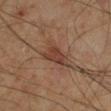A male patient, approximately 70 years of age.
Cropped from a total-body skin-imaging series; the visible field is about 15 mm.
This is a cross-polarized tile.
The lesion is located on the leg.
An algorithmic analysis of the crop reported an outline eccentricity of about 0.9 (0 = round, 1 = elongated). The software also gave border irregularity of about 4.5 on a 0–10 scale and radial color variation of about 1. And it measured a nevus-likeness score of about 15/100 and a detector confidence of about 100 out of 100 that the crop contains a lesion.